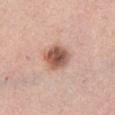A female subject, in their mid-60s.
Cropped from a whole-body photographic skin survey; the tile spans about 15 mm.
The lesion-visualizer software estimated an area of roughly 9.5 mm² and an outline eccentricity of about 0.6 (0 = round, 1 = elongated). The analysis additionally found internal color variation of about 6.5 on a 0–10 scale and a peripheral color-asymmetry measure near 2.
The lesion is located on the right thigh.
The lesion's longest dimension is about 4 mm.